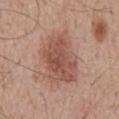Clinical impression:
Imaged during a routine full-body skin examination; the lesion was not biopsied and no histopathology is available.
Clinical summary:
Captured under white-light illumination. From the mid back. The total-body-photography lesion software estimated an area of roughly 24 mm² and an eccentricity of roughly 0.8. It also reported an automated nevus-likeness rating near 90 out of 100. Approximately 7 mm at its widest. A male patient about 55 years old. A 15 mm crop from a total-body photograph taken for skin-cancer surveillance.